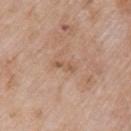<record>
<biopsy_status>not biopsied; imaged during a skin examination</biopsy_status>
<image>
  <source>total-body photography crop</source>
  <field_of_view_mm>15</field_of_view_mm>
</image>
<lighting>white-light</lighting>
<automated_metrics>
  <area_mm2_approx>2.5</area_mm2_approx>
  <eccentricity>0.9</eccentricity>
  <shape_asymmetry>0.3</shape_asymmetry>
  <vs_skin_darker_L>7.0</vs_skin_darker_L>
  <vs_skin_contrast_norm>5.5</vs_skin_contrast_norm>
  <nevus_likeness_0_100>0</nevus_likeness_0_100>
  <lesion_detection_confidence_0_100>100</lesion_detection_confidence_0_100>
</automated_metrics>
<patient>
  <sex>female</sex>
  <age_approx>70</age_approx>
</patient>
<lesion_size>
  <long_diameter_mm_approx>3.0</long_diameter_mm_approx>
</lesion_size>
<site>arm</site>
</record>